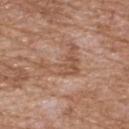No biopsy was performed on this lesion — it was imaged during a full skin examination and was not determined to be concerning.
The lesion is located on the chest.
Imaged with white-light lighting.
A male subject aged approximately 60.
The recorded lesion diameter is about 5 mm.
A region of skin cropped from a whole-body photographic capture, roughly 15 mm wide.